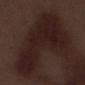Imaged during a routine full-body skin examination; the lesion was not biopsied and no histopathology is available.
A male patient approximately 70 years of age.
From the left thigh.
A lesion tile, about 15 mm wide, cut from a 3D total-body photograph.
Approximately 14 mm at its widest.